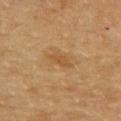Located on the upper back. A close-up tile cropped from a whole-body skin photograph, about 15 mm across. The lesion's longest dimension is about 3 mm. The patient is a female aged 58 to 62. Imaged with cross-polarized lighting.